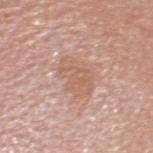The lesion was tiled from a total-body skin photograph and was not biopsied. Captured under white-light illumination. Located on the head or neck. Cropped from a whole-body photographic skin survey; the tile spans about 15 mm. The subject is a male aged around 60. Automated tile analysis of the lesion measured an area of roughly 10 mm², an eccentricity of roughly 0.75, and two-axis asymmetry of about 0.25. And it measured a lesion color around L≈61 a*≈19 b*≈29 in CIELAB and a normalized border contrast of about 5. It also reported a border-irregularity rating of about 3/10 and a color-variation rating of about 2/10.